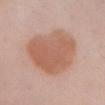The lesion was photographed on a routine skin check and not biopsied; there is no pathology result.
The lesion is on the chest.
A 15 mm crop from a total-body photograph taken for skin-cancer surveillance.
A female patient aged around 40.